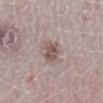image source = 15 mm crop, total-body photography; patient = female, aged approximately 50; site = the right lower leg.